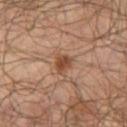Recorded during total-body skin imaging; not selected for excision or biopsy. Longest diameter approximately 2.5 mm. This is a cross-polarized tile. A male patient in their mid-60s. A roughly 15 mm field-of-view crop from a total-body skin photograph. From the right thigh.